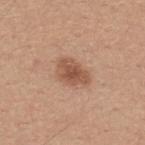Captured during whole-body skin photography for melanoma surveillance; the lesion was not biopsied.
This image is a 15 mm lesion crop taken from a total-body photograph.
The recorded lesion diameter is about 4 mm.
A male patient about 30 years old.
The lesion is located on the upper back.